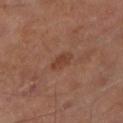Image and clinical context: Located on the leg. A male subject approximately 70 years of age. A region of skin cropped from a whole-body photographic capture, roughly 15 mm wide.